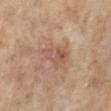The lesion is on the right leg.
A roughly 15 mm field-of-view crop from a total-body skin photograph.
The patient is a female roughly 65 years of age.